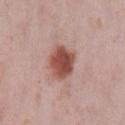patient: female, aged around 40; image: 15 mm crop, total-body photography; illumination: white-light illumination; location: the left lower leg; lesion size: ≈4 mm.